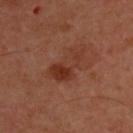The lesion-visualizer software estimated an area of roughly 10 mm², an eccentricity of roughly 0.85, and a symmetry-axis asymmetry near 0.4. The analysis additionally found a lesion color around L≈34 a*≈25 b*≈29 in CIELAB, roughly 7 lightness units darker than nearby skin, and a normalized border contrast of about 6.5. The analysis additionally found a lesion-detection confidence of about 100/100. Captured under cross-polarized illumination. A male patient aged 48 to 52. A 15 mm close-up extracted from a 3D total-body photography capture. The lesion's longest dimension is about 5.5 mm. The lesion is on the upper back.